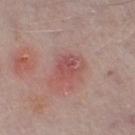This lesion was catalogued during total-body skin photography and was not selected for biopsy. An algorithmic analysis of the crop reported an area of roughly 5.5 mm², an outline eccentricity of about 0.6 (0 = round, 1 = elongated), and two-axis asymmetry of about 0.3. The software also gave a nevus-likeness score of about 5/100 and a detector confidence of about 100 out of 100 that the crop contains a lesion. This is a white-light tile. This image is a 15 mm lesion crop taken from a total-body photograph. From the left thigh. The subject is a male aged 73–77. Longest diameter approximately 2.5 mm.